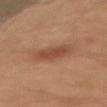notes: catalogued during a skin exam; not biopsied
patient: male, in their mid-40s
acquisition: total-body-photography crop, ~15 mm field of view
automated metrics: a shape eccentricity near 0.6; a mean CIELAB color near L≈46 a*≈23 b*≈30, roughly 8 lightness units darker than nearby skin, and a normalized border contrast of about 6.5; border irregularity of about 2 on a 0–10 scale, a within-lesion color-variation index near 2.5/10, and radial color variation of about 1; lesion-presence confidence of about 100/100
diameter: ≈3.5 mm
anatomic site: the arm
illumination: cross-polarized